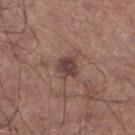<case>
<biopsy_status>not biopsied; imaged during a skin examination</biopsy_status>
<lighting>white-light</lighting>
<automated_metrics>
  <cielab_L>42</cielab_L>
  <cielab_a>18</cielab_a>
  <cielab_b>20</cielab_b>
  <vs_skin_darker_L>10.0</vs_skin_darker_L>
  <vs_skin_contrast_norm>8.5</vs_skin_contrast_norm>
  <border_irregularity_0_10>3.0</border_irregularity_0_10>
  <color_variation_0_10>3.0</color_variation_0_10>
  <peripheral_color_asymmetry>1.0</peripheral_color_asymmetry>
  <nevus_likeness_0_100>5</nevus_likeness_0_100>
  <lesion_detection_confidence_0_100>100</lesion_detection_confidence_0_100>
</automated_metrics>
<site>left lower leg</site>
<image>
  <source>total-body photography crop</source>
  <field_of_view_mm>15</field_of_view_mm>
</image>
<lesion_size>
  <long_diameter_mm_approx>3.0</long_diameter_mm_approx>
</lesion_size>
<patient>
  <sex>male</sex>
  <age_approx>60</age_approx>
</patient>
</case>